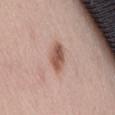<record>
  <image>
    <source>total-body photography crop</source>
    <field_of_view_mm>15</field_of_view_mm>
  </image>
  <lighting>white-light</lighting>
  <lesion_size>
    <long_diameter_mm_approx>3.5</long_diameter_mm_approx>
  </lesion_size>
  <site>lower back</site>
  <patient>
    <sex>male</sex>
    <age_approx>55</age_approx>
  </patient>
</record>